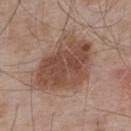Imaged during a routine full-body skin examination; the lesion was not biopsied and no histopathology is available. A male patient approximately 55 years of age. The total-body-photography lesion software estimated a mean CIELAB color near L≈48 a*≈19 b*≈27, a lesion–skin lightness drop of about 11, and a normalized border contrast of about 8.5. The software also gave border irregularity of about 2.5 on a 0–10 scale and a color-variation rating of about 5/10. Imaged with white-light lighting. The lesion is on the upper back. This image is a 15 mm lesion crop taken from a total-body photograph.